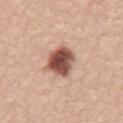The lesion was photographed on a routine skin check and not biopsied; there is no pathology result. A lesion tile, about 15 mm wide, cut from a 3D total-body photograph. From the lower back. The patient is a male in their mid- to late 50s. Imaged with white-light lighting. Measured at roughly 4 mm in maximum diameter.